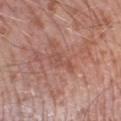Captured under white-light illumination.
Measured at roughly 3.5 mm in maximum diameter.
The patient is a male aged 73–77.
From the left lower leg.
Cropped from a total-body skin-imaging series; the visible field is about 15 mm.